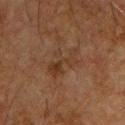The lesion was tiled from a total-body skin photograph and was not biopsied. The patient is a male roughly 65 years of age. On the chest. A 15 mm close-up tile from a total-body photography series done for melanoma screening. The lesion's longest dimension is about 4.5 mm.